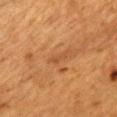Part of a total-body skin-imaging series; this lesion was reviewed on a skin check and was not flagged for biopsy. On the upper back. A 15 mm close-up tile from a total-body photography series done for melanoma screening. The patient is a female aged around 55. This is a cross-polarized tile.